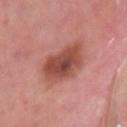The lesion was photographed on a routine skin check and not biopsied; there is no pathology result.
A close-up tile cropped from a whole-body skin photograph, about 15 mm across.
A male subject, in their 40s.
The total-body-photography lesion software estimated a lesion area of about 18 mm² and a shape-asymmetry score of about 0.15 (0 = symmetric).
Captured under white-light illumination.
About 5.5 mm across.
The lesion is on the right lower leg.